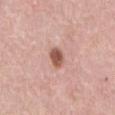Q: Lesion size?
A: about 3 mm
Q: Who is the patient?
A: female, approximately 65 years of age
Q: What is the imaging modality?
A: ~15 mm tile from a whole-body skin photo
Q: What lighting was used for the tile?
A: white-light illumination
Q: Where on the body is the lesion?
A: the front of the torso
Q: Automated lesion metrics?
A: a mean CIELAB color near L≈55 a*≈23 b*≈27, roughly 14 lightness units darker than nearby skin, and a normalized border contrast of about 9; a border-irregularity index near 1.5/10, a within-lesion color-variation index near 3.5/10, and a peripheral color-asymmetry measure near 1; a classifier nevus-likeness of about 90/100 and a lesion-detection confidence of about 100/100